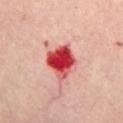Notes:
- biopsy status · total-body-photography surveillance lesion; no biopsy
- acquisition · ~15 mm tile from a whole-body skin photo
- body site · the chest
- subject · female, in their 60s